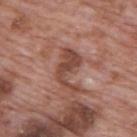Assessment:
Part of a total-body skin-imaging series; this lesion was reviewed on a skin check and was not flagged for biopsy.
Context:
Located on the upper back. A close-up tile cropped from a whole-body skin photograph, about 15 mm across. An algorithmic analysis of the crop reported an area of roughly 8 mm², a shape eccentricity near 0.85, and a symmetry-axis asymmetry near 0.6. It also reported a border-irregularity index near 8.5/10 and a peripheral color-asymmetry measure near 0.5. And it measured a detector confidence of about 95 out of 100 that the crop contains a lesion. A male patient in their 70s.